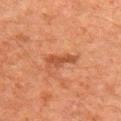biopsy status: no biopsy performed (imaged during a skin exam)
illumination: cross-polarized illumination
subject: male, aged 58 to 62
anatomic site: the right upper arm
imaging modality: ~15 mm tile from a whole-body skin photo